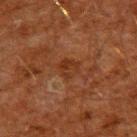Assessment: Part of a total-body skin-imaging series; this lesion was reviewed on a skin check and was not flagged for biopsy. Image and clinical context: Cropped from a total-body skin-imaging series; the visible field is about 15 mm. Imaged with cross-polarized lighting. A male subject roughly 60 years of age. The lesion is located on the upper back. Longest diameter approximately 2.5 mm.